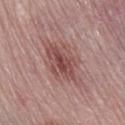  biopsy_status: not biopsied; imaged during a skin examination
  site: right thigh
  patient:
    sex: female
    age_approx: 65
  image:
    source: total-body photography crop
    field_of_view_mm: 15
  automated_metrics:
    area_mm2_approx: 18.0
    eccentricity: 0.85
    shape_asymmetry: 0.25
    color_variation_0_10: 6.0
    peripheral_color_asymmetry: 2.0
  lighting: white-light